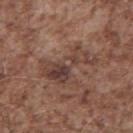{"biopsy_status": "not biopsied; imaged during a skin examination", "automated_metrics": {"area_mm2_approx": 13.0, "eccentricity": 0.85, "shape_asymmetry": 0.6, "nevus_likeness_0_100": 0, "lesion_detection_confidence_0_100": 90}, "site": "mid back", "lighting": "white-light", "image": {"source": "total-body photography crop", "field_of_view_mm": 15}, "lesion_size": {"long_diameter_mm_approx": 6.5}, "patient": {"sex": "male", "age_approx": 55}}